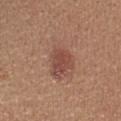No biopsy was performed on this lesion — it was imaged during a full skin examination and was not determined to be concerning.
This is a white-light tile.
Measured at roughly 5.5 mm in maximum diameter.
The lesion is on the head or neck.
The patient is a female aged 38 to 42.
Automated image analysis of the tile measured a classifier nevus-likeness of about 85/100 and a lesion-detection confidence of about 100/100.
A close-up tile cropped from a whole-body skin photograph, about 15 mm across.